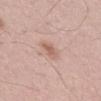Recorded during total-body skin imaging; not selected for excision or biopsy.
The total-body-photography lesion software estimated roughly 9 lightness units darker than nearby skin and a normalized border contrast of about 6.5. The software also gave an automated nevus-likeness rating near 15 out of 100.
Captured under white-light illumination.
From the front of the torso.
A male patient in their mid-50s.
A lesion tile, about 15 mm wide, cut from a 3D total-body photograph.